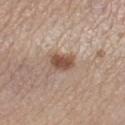Part of a total-body skin-imaging series; this lesion was reviewed on a skin check and was not flagged for biopsy. A female patient roughly 65 years of age. The lesion is on the right lower leg. This image is a 15 mm lesion crop taken from a total-body photograph.